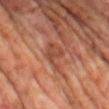Assessment: The lesion was photographed on a routine skin check and not biopsied; there is no pathology result. Clinical summary: A 15 mm crop from a total-body photograph taken for skin-cancer surveillance. An algorithmic analysis of the crop reported an eccentricity of roughly 0.75. The software also gave a border-irregularity rating of about 5/10, internal color variation of about 2.5 on a 0–10 scale, and peripheral color asymmetry of about 1. On the upper back. The patient is a male aged 58–62.